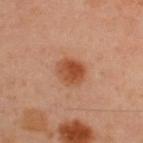Imaged during a routine full-body skin examination; the lesion was not biopsied and no histopathology is available. A male patient about 45 years old. The tile uses cross-polarized illumination. Approximately 3.5 mm at its widest. A 15 mm close-up tile from a total-body photography series done for melanoma screening. The total-body-photography lesion software estimated an area of roughly 8 mm² and a shape eccentricity near 0.55. And it measured a border-irregularity rating of about 1.5/10 and a within-lesion color-variation index near 5.5/10. The lesion is located on the upper back.